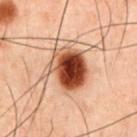Impression: This lesion was catalogued during total-body skin photography and was not selected for biopsy. Background: This is a cross-polarized tile. From the chest. An algorithmic analysis of the crop reported a symmetry-axis asymmetry near 0.2. The software also gave a lesion–skin lightness drop of about 18 and a normalized border contrast of about 14.5. A 15 mm close-up extracted from a 3D total-body photography capture. A male subject, aged 58 to 62.